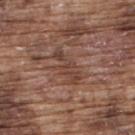Assessment: Captured during whole-body skin photography for melanoma surveillance; the lesion was not biopsied. Context: The total-body-photography lesion software estimated roughly 7 lightness units darker than nearby skin. The software also gave border irregularity of about 6 on a 0–10 scale, a within-lesion color-variation index near 2.5/10, and a peripheral color-asymmetry measure near 1. From the upper back. A male subject, approximately 75 years of age. A 15 mm crop from a total-body photograph taken for skin-cancer surveillance.